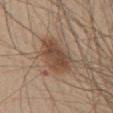• follow-up — imaged on a skin check; not biopsied
• image source — ~15 mm tile from a whole-body skin photo
• patient — male, aged approximately 60
• anatomic site — the chest
• size — ≈4.5 mm
• tile lighting — white-light illumination